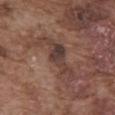This lesion was catalogued during total-body skin photography and was not selected for biopsy. A male subject aged 73–77. A 15 mm close-up extracted from a 3D total-body photography capture. Located on the abdomen.